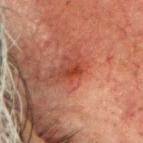Q: Was this lesion biopsied?
A: catalogued during a skin exam; not biopsied
Q: Patient demographics?
A: male, aged approximately 80
Q: What kind of image is this?
A: total-body-photography crop, ~15 mm field of view
Q: Where on the body is the lesion?
A: the head or neck